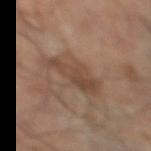The recorded lesion diameter is about 5.5 mm. This image is a 15 mm lesion crop taken from a total-body photograph. The lesion is on the leg. The lesion-visualizer software estimated a detector confidence of about 100 out of 100 that the crop contains a lesion.